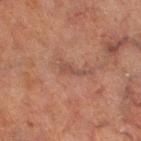This lesion was catalogued during total-body skin photography and was not selected for biopsy. Located on the left thigh. The subject is a male aged 63 to 67. A lesion tile, about 15 mm wide, cut from a 3D total-body photograph.